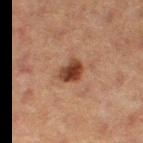No biopsy was performed on this lesion — it was imaged during a full skin examination and was not determined to be concerning. A female subject aged 38 to 42. This image is a 15 mm lesion crop taken from a total-body photograph. The recorded lesion diameter is about 3 mm. On the right thigh. An algorithmic analysis of the crop reported a within-lesion color-variation index near 6/10 and a peripheral color-asymmetry measure near 2. The software also gave an automated nevus-likeness rating near 95 out of 100 and a lesion-detection confidence of about 100/100. This is a cross-polarized tile.